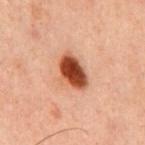The lesion was tiled from a total-body skin photograph and was not biopsied. Imaged with cross-polarized lighting. About 4 mm across. A close-up tile cropped from a whole-body skin photograph, about 15 mm across. Automated image analysis of the tile measured a lesion color around L≈37 a*≈23 b*≈29 in CIELAB, a lesion–skin lightness drop of about 17, and a lesion-to-skin contrast of about 13.5 (normalized; higher = more distinct). The software also gave border irregularity of about 2.5 on a 0–10 scale, internal color variation of about 5.5 on a 0–10 scale, and a peripheral color-asymmetry measure near 1.5. It also reported lesion-presence confidence of about 100/100. The patient is a male roughly 60 years of age. Located on the chest.